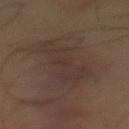Captured during whole-body skin photography for melanoma surveillance; the lesion was not biopsied.
A 15 mm crop from a total-body photograph taken for skin-cancer surveillance.
Located on the front of the torso.
A male patient roughly 55 years of age.
Automated image analysis of the tile measured an area of roughly 20 mm², an outline eccentricity of about 0.9 (0 = round, 1 = elongated), and two-axis asymmetry of about 0.4. It also reported a lesion color around L≈32 a*≈13 b*≈19 in CIELAB, a lesion–skin lightness drop of about 5, and a normalized border contrast of about 5.5. It also reported a border-irregularity index near 6.5/10, internal color variation of about 3.5 on a 0–10 scale, and a peripheral color-asymmetry measure near 1.5. The software also gave a nevus-likeness score of about 0/100 and a detector confidence of about 90 out of 100 that the crop contains a lesion.
The recorded lesion diameter is about 8 mm.